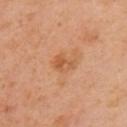Imaged during a routine full-body skin examination; the lesion was not biopsied and no histopathology is available.
On the left arm.
A female patient, aged approximately 40.
A region of skin cropped from a whole-body photographic capture, roughly 15 mm wide.